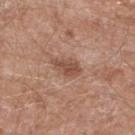| key | value |
|---|---|
| follow-up | catalogued during a skin exam; not biopsied |
| automated lesion analysis | a footprint of about 5.5 mm², an eccentricity of roughly 0.8, and a shape-asymmetry score of about 0.25 (0 = symmetric); a lesion–skin lightness drop of about 10 and a normalized border contrast of about 7; a within-lesion color-variation index near 2.5/10 and peripheral color asymmetry of about 1; a classifier nevus-likeness of about 40/100 and lesion-presence confidence of about 100/100 |
| diameter | ~3.5 mm (longest diameter) |
| patient | male, about 60 years old |
| image | 15 mm crop, total-body photography |
| location | the leg |
| illumination | white-light |